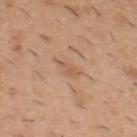* biopsy status — catalogued during a skin exam; not biopsied
* site — the upper back
* patient — male, aged 38 to 42
* image source — ~15 mm tile from a whole-body skin photo
* lesion size — ≈2.5 mm
* automated lesion analysis — an area of roughly 2.5 mm² and an outline eccentricity of about 0.9 (0 = round, 1 = elongated); a border-irregularity rating of about 4/10 and a within-lesion color-variation index near 0/10